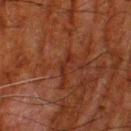{"biopsy_status": "not biopsied; imaged during a skin examination", "lighting": "cross-polarized", "site": "left thigh", "lesion_size": {"long_diameter_mm_approx": 3.0}, "image": {"source": "total-body photography crop", "field_of_view_mm": 15}, "patient": {"sex": "male", "age_approx": 80}}